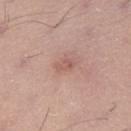Clinical summary:
Located on the left lower leg. Cropped from a whole-body photographic skin survey; the tile spans about 15 mm. A male patient aged 33–37.